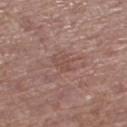| feature | finding |
|---|---|
| subject | female, aged around 75 |
| image | total-body-photography crop, ~15 mm field of view |
| anatomic site | the leg |